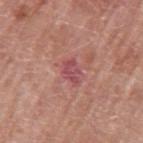Assessment: Recorded during total-body skin imaging; not selected for excision or biopsy. Background: Automated tile analysis of the lesion measured an area of roughly 4.5 mm², an eccentricity of roughly 0.75, and two-axis asymmetry of about 0.25. Measured at roughly 3 mm in maximum diameter. A female patient, aged 58 to 62. The tile uses white-light illumination. A 15 mm close-up tile from a total-body photography series done for melanoma screening. The lesion is on the left upper arm.